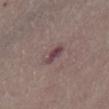{
  "site": "front of the torso",
  "patient": {
    "sex": "male",
    "age_approx": 75
  },
  "automated_metrics": {
    "color_variation_0_10": 1.5,
    "peripheral_color_asymmetry": 0.5,
    "nevus_likeness_0_100": 0
  },
  "image": {
    "source": "total-body photography crop",
    "field_of_view_mm": 15
  },
  "lesion_size": {
    "long_diameter_mm_approx": 3.0
  },
  "lighting": "white-light"
}